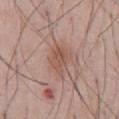Notes:
– notes — no biopsy performed (imaged during a skin exam)
– patient — male, aged around 55
– image-analysis metrics — an average lesion color of about L≈53 a*≈21 b*≈27 (CIELAB) and roughly 8 lightness units darker than nearby skin
– location — the abdomen
– acquisition — ~15 mm tile from a whole-body skin photo
– lesion diameter — ~3.5 mm (longest diameter)
– lighting — white-light illumination